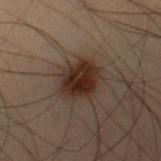Part of a total-body skin-imaging series; this lesion was reviewed on a skin check and was not flagged for biopsy.
A 15 mm close-up extracted from a 3D total-body photography capture.
The lesion is on the right thigh.
A male patient, aged around 55.